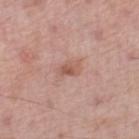Imaged during a routine full-body skin examination; the lesion was not biopsied and no histopathology is available. From the right lower leg. A 15 mm close-up tile from a total-body photography series done for melanoma screening. The patient is a male aged 68 to 72. This is a white-light tile.